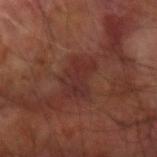| key | value |
|---|---|
| biopsy status | imaged on a skin check; not biopsied |
| patient | male, aged 58–62 |
| imaging modality | ~15 mm tile from a whole-body skin photo |
| illumination | cross-polarized illumination |
| location | the right arm |
| size | ≈4 mm |
| image-analysis metrics | a footprint of about 9 mm² and a shape-asymmetry score of about 0.4 (0 = symmetric); a mean CIELAB color near L≈29 a*≈23 b*≈22, roughly 5 lightness units darker than nearby skin, and a lesion-to-skin contrast of about 6 (normalized; higher = more distinct); a border-irregularity index near 7/10 and peripheral color asymmetry of about 1; a nevus-likeness score of about 0/100 and lesion-presence confidence of about 100/100 |